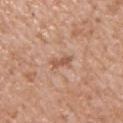• workup · total-body-photography surveillance lesion; no biopsy
• diameter · ~2.5 mm (longest diameter)
• body site · the chest
• image · total-body-photography crop, ~15 mm field of view
• automated metrics · a lesion area of about 3 mm² and a shape eccentricity near 0.9; a lesion color around L≈56 a*≈22 b*≈31 in CIELAB and a normalized border contrast of about 6.5; a classifier nevus-likeness of about 0/100
• subject · male, aged around 60
• tile lighting · white-light